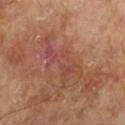notes — imaged on a skin check; not biopsied
lesion size — ~7 mm (longest diameter)
subject — male, about 65 years old
automated metrics — an outline eccentricity of about 0.9 (0 = round, 1 = elongated) and a symmetry-axis asymmetry near 0.55; a border-irregularity rating of about 9/10 and internal color variation of about 4 on a 0–10 scale
lighting — cross-polarized illumination
acquisition — ~15 mm crop, total-body skin-cancer survey